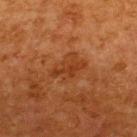follow-up = no biopsy performed (imaged during a skin exam) | automated metrics = a normalized lesion–skin contrast near 5.5; peripheral color asymmetry of about 1; a nevus-likeness score of about 0/100 | lighting = cross-polarized | subject = male, aged 63–67 | size = ~4 mm (longest diameter) | body site = the upper back | image source = total-body-photography crop, ~15 mm field of view.